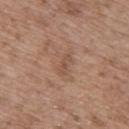{
  "patient": {
    "sex": "male",
    "age_approx": 70
  },
  "lighting": "white-light",
  "image": {
    "source": "total-body photography crop",
    "field_of_view_mm": 15
  },
  "automated_metrics": {
    "area_mm2_approx": 2.0,
    "eccentricity": 0.95,
    "shape_asymmetry": 0.4,
    "border_irregularity_0_10": 5.0,
    "color_variation_0_10": 0.0,
    "peripheral_color_asymmetry": 0.0
  },
  "lesion_size": {
    "long_diameter_mm_approx": 2.5
  },
  "site": "mid back"
}